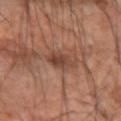Imaged during a routine full-body skin examination; the lesion was not biopsied and no histopathology is available. On the left forearm. The subject is a male aged around 60. A roughly 15 mm field-of-view crop from a total-body skin photograph.